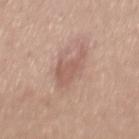Impression: Imaged during a routine full-body skin examination; the lesion was not biopsied and no histopathology is available. Clinical summary: Automated image analysis of the tile measured a lesion color around L≈57 a*≈20 b*≈26 in CIELAB and about 8 CIELAB-L* units darker than the surrounding skin. It also reported a lesion-detection confidence of about 100/100. From the mid back. The tile uses white-light illumination. A male patient, aged approximately 30. About 4 mm across. Cropped from a total-body skin-imaging series; the visible field is about 15 mm.